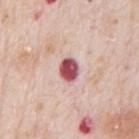Impression: The lesion was tiled from a total-body skin photograph and was not biopsied. Clinical summary: A lesion tile, about 15 mm wide, cut from a 3D total-body photograph. Approximately 2.5 mm at its widest. A male patient, in their 80s. On the chest.